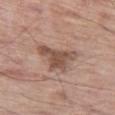Recorded during total-body skin imaging; not selected for excision or biopsy. The lesion-visualizer software estimated a lesion color around L≈51 a*≈18 b*≈26 in CIELAB, roughly 11 lightness units darker than nearby skin, and a normalized border contrast of about 8. And it measured internal color variation of about 3 on a 0–10 scale. The recorded lesion diameter is about 4.5 mm. A male subject, approximately 70 years of age. On the left thigh. A close-up tile cropped from a whole-body skin photograph, about 15 mm across.